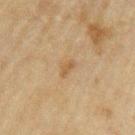workup: imaged on a skin check; not biopsied
anatomic site: the left upper arm
patient: male, about 70 years old
image: ~15 mm tile from a whole-body skin photo